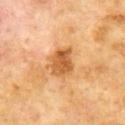Q: Is there a histopathology result?
A: total-body-photography surveillance lesion; no biopsy
Q: Where on the body is the lesion?
A: the chest
Q: What is the imaging modality?
A: 15 mm crop, total-body photography
Q: Patient demographics?
A: male, about 60 years old